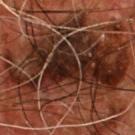Captured during whole-body skin photography for melanoma surveillance; the lesion was not biopsied.
A 15 mm close-up extracted from a 3D total-body photography capture.
On the front of the torso.
A male patient, aged 53–57.
The tile uses cross-polarized illumination.
The recorded lesion diameter is about 14 mm.